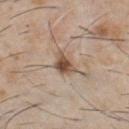Clinical impression:
No biopsy was performed on this lesion — it was imaged during a full skin examination and was not determined to be concerning.
Clinical summary:
Automated tile analysis of the lesion measured a lesion area of about 5.5 mm², a shape eccentricity near 0.65, and two-axis asymmetry of about 0.3. The software also gave a mean CIELAB color near L≈51 a*≈16 b*≈28, a lesion–skin lightness drop of about 14, and a normalized border contrast of about 9.5. Cropped from a total-body skin-imaging series; the visible field is about 15 mm. Approximately 3 mm at its widest. From the chest. The patient is a male in their 60s. This is a white-light tile.